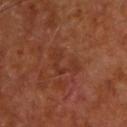Q: What is the imaging modality?
A: total-body-photography crop, ~15 mm field of view
Q: Who is the patient?
A: male, roughly 65 years of age
Q: What lighting was used for the tile?
A: cross-polarized illumination
Q: Lesion location?
A: the upper back
Q: What did automated image analysis measure?
A: a lesion area of about 6 mm² and a shape-asymmetry score of about 0.7 (0 = symmetric); a mean CIELAB color near L≈32 a*≈24 b*≈29, roughly 5 lightness units darker than nearby skin, and a lesion-to-skin contrast of about 4.5 (normalized; higher = more distinct)